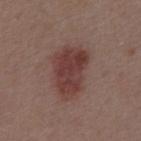notes=imaged on a skin check; not biopsied
diameter=≈6 mm
lighting=white-light
image=~15 mm crop, total-body skin-cancer survey
patient=male, roughly 55 years of age
automated metrics=about 10 CIELAB-L* units darker than the surrounding skin and a normalized lesion–skin contrast near 8.5
location=the abdomen